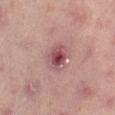{"biopsy_status": "not biopsied; imaged during a skin examination", "lesion_size": {"long_diameter_mm_approx": 4.0}, "patient": {"sex": "female", "age_approx": 50}, "site": "leg", "lighting": "cross-polarized", "image": {"source": "total-body photography crop", "field_of_view_mm": 15}, "automated_metrics": {"border_irregularity_0_10": 1.5, "color_variation_0_10": 8.5, "lesion_detection_confidence_0_100": 100}}